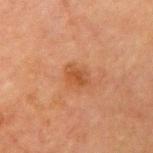Clinical impression: No biopsy was performed on this lesion — it was imaged during a full skin examination and was not determined to be concerning. Context: A male subject aged 63 to 67. The lesion-visualizer software estimated a footprint of about 4.5 mm² and an eccentricity of roughly 0.65. The analysis additionally found a lesion color around L≈41 a*≈22 b*≈33 in CIELAB, roughly 7 lightness units darker than nearby skin, and a lesion-to-skin contrast of about 6.5 (normalized; higher = more distinct). A lesion tile, about 15 mm wide, cut from a 3D total-body photograph. Imaged with cross-polarized lighting. Measured at roughly 3 mm in maximum diameter. The lesion is located on the left upper arm.